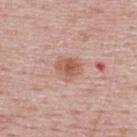Q: Is there a histopathology result?
A: no biopsy performed (imaged during a skin exam)
Q: Lesion size?
A: ≈3 mm
Q: What kind of image is this?
A: ~15 mm tile from a whole-body skin photo
Q: What is the anatomic site?
A: the upper back
Q: What are the patient's age and sex?
A: male, aged 48–52
Q: What lighting was used for the tile?
A: white-light